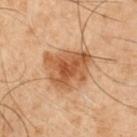Clinical impression: Part of a total-body skin-imaging series; this lesion was reviewed on a skin check and was not flagged for biopsy. Acquisition and patient details: A male subject, about 50 years old. An algorithmic analysis of the crop reported a lesion color around L≈42 a*≈18 b*≈30 in CIELAB, about 10 CIELAB-L* units darker than the surrounding skin, and a normalized lesion–skin contrast near 8.5. The software also gave a border-irregularity index near 3/10, a color-variation rating of about 5/10, and a peripheral color-asymmetry measure near 1.5. Longest diameter approximately 6 mm. The lesion is located on the right upper arm. A roughly 15 mm field-of-view crop from a total-body skin photograph.